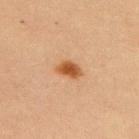The lesion was photographed on a routine skin check and not biopsied; there is no pathology result. The patient is a male in their 60s. The lesion is on the upper back. The total-body-photography lesion software estimated an area of roughly 4.5 mm² and a shape eccentricity near 0.75. And it measured border irregularity of about 2 on a 0–10 scale, a within-lesion color-variation index near 3/10, and a peripheral color-asymmetry measure near 1. And it measured an automated nevus-likeness rating near 100 out of 100 and lesion-presence confidence of about 100/100. The tile uses cross-polarized illumination. Measured at roughly 3 mm in maximum diameter. A 15 mm crop from a total-body photograph taken for skin-cancer surveillance.